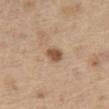follow-up=no biopsy performed (imaged during a skin exam) | image source=~15 mm crop, total-body skin-cancer survey | lesion size=~2.5 mm (longest diameter) | body site=the leg | subject=male, approximately 70 years of age | TBP lesion metrics=a lesion area of about 4 mm² and a symmetry-axis asymmetry near 0.25; a classifier nevus-likeness of about 90/100 and a lesion-detection confidence of about 100/100.